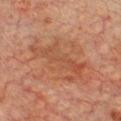| key | value |
|---|---|
| follow-up | catalogued during a skin exam; not biopsied |
| image | 15 mm crop, total-body photography |
| subject | male, aged 73 to 77 |
| anatomic site | the chest |